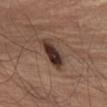This lesion was catalogued during total-body skin photography and was not selected for biopsy. Measured at roughly 4.5 mm in maximum diameter. The lesion is located on the left thigh. A close-up tile cropped from a whole-body skin photograph, about 15 mm across. A female subject approximately 65 years of age. The tile uses white-light illumination.